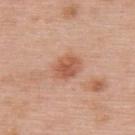Q: Is there a histopathology result?
A: no biopsy performed (imaged during a skin exam)
Q: Patient demographics?
A: male, aged approximately 60
Q: Where on the body is the lesion?
A: the upper back
Q: How was this image acquired?
A: total-body-photography crop, ~15 mm field of view
Q: What lighting was used for the tile?
A: white-light
Q: How large is the lesion?
A: about 3.5 mm